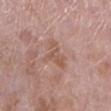<record>
  <biopsy_status>not biopsied; imaged during a skin examination</biopsy_status>
  <lesion_size>
    <long_diameter_mm_approx>4.0</long_diameter_mm_approx>
  </lesion_size>
  <patient>
    <sex>female</sex>
    <age_approx>70</age_approx>
  </patient>
  <lighting>white-light</lighting>
  <site>left lower leg</site>
  <image>
    <source>total-body photography crop</source>
    <field_of_view_mm>15</field_of_view_mm>
  </image>
</record>